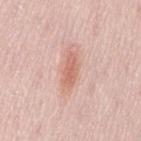workup: no biopsy performed (imaged during a skin exam)
tile lighting: white-light illumination
acquisition: total-body-photography crop, ~15 mm field of view
location: the lower back
TBP lesion metrics: a lesion area of about 6 mm² and an eccentricity of roughly 0.9; border irregularity of about 3 on a 0–10 scale and internal color variation of about 2.5 on a 0–10 scale; a classifier nevus-likeness of about 45/100
lesion diameter: ~4 mm (longest diameter)
subject: female, approximately 50 years of age